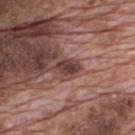Imaged during a routine full-body skin examination; the lesion was not biopsied and no histopathology is available.
The lesion-visualizer software estimated a footprint of about 3.5 mm², an eccentricity of roughly 0.85, and a shape-asymmetry score of about 0.25 (0 = symmetric). The software also gave a border-irregularity index near 2.5/10 and a peripheral color-asymmetry measure near 1. The analysis additionally found a classifier nevus-likeness of about 0/100 and a detector confidence of about 100 out of 100 that the crop contains a lesion.
A male patient roughly 70 years of age.
The lesion's longest dimension is about 3 mm.
On the upper back.
A roughly 15 mm field-of-view crop from a total-body skin photograph.